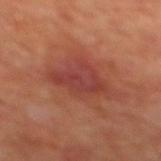  biopsy_status: not biopsied; imaged during a skin examination
  site: back
  patient:
    sex: male
    age_approx: 70
  image:
    source: total-body photography crop
    field_of_view_mm: 15